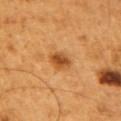* biopsy status — total-body-photography surveillance lesion; no biopsy
* lesion diameter — about 3 mm
* illumination — cross-polarized
* acquisition — 15 mm crop, total-body photography
* patient — male, aged approximately 60
* site — the left upper arm
* image-analysis metrics — a mean CIELAB color near L≈51 a*≈27 b*≈44, about 13 CIELAB-L* units darker than the surrounding skin, and a lesion-to-skin contrast of about 8.5 (normalized; higher = more distinct)